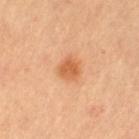Clinical impression:
The lesion was tiled from a total-body skin photograph and was not biopsied.
Acquisition and patient details:
On the left thigh. A female patient, aged approximately 60. The tile uses cross-polarized illumination. The recorded lesion diameter is about 2.5 mm. This image is a 15 mm lesion crop taken from a total-body photograph.